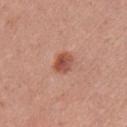biopsy status = catalogued during a skin exam; not biopsied
location = the chest
diameter = ≈2.5 mm
subject = male, about 25 years old
illumination = white-light
acquisition = 15 mm crop, total-body photography